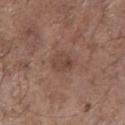Clinical impression: Imaged during a routine full-body skin examination; the lesion was not biopsied and no histopathology is available. Acquisition and patient details: Measured at roughly 3 mm in maximum diameter. A 15 mm close-up extracted from a 3D total-body photography capture. The subject is a male about 70 years old. Captured under white-light illumination. From the chest.